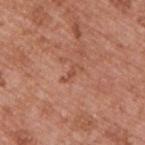The lesion was photographed on a routine skin check and not biopsied; there is no pathology result. The lesion is located on the back. Longest diameter approximately 2.5 mm. This image is a 15 mm lesion crop taken from a total-body photograph. The tile uses white-light illumination. A male patient, aged approximately 55. Automated image analysis of the tile measured a nevus-likeness score of about 0/100.